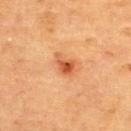{"biopsy_status": "not biopsied; imaged during a skin examination", "site": "upper back", "lesion_size": {"long_diameter_mm_approx": 3.0}, "patient": {"sex": "female", "age_approx": 70}, "image": {"source": "total-body photography crop", "field_of_view_mm": 15}, "lighting": "cross-polarized", "automated_metrics": {"border_irregularity_0_10": 3.5, "color_variation_0_10": 4.5, "peripheral_color_asymmetry": 1.5}}